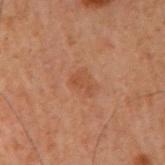workup: no biopsy performed (imaged during a skin exam) | illumination: cross-polarized illumination | anatomic site: the right upper arm | acquisition: total-body-photography crop, ~15 mm field of view | lesion diameter: ~2.5 mm (longest diameter) | image-analysis metrics: a mean CIELAB color near L≈37 a*≈20 b*≈27, about 5 CIELAB-L* units darker than the surrounding skin, and a lesion-to-skin contrast of about 5 (normalized; higher = more distinct); a nevus-likeness score of about 0/100 and a lesion-detection confidence of about 100/100 | patient: male, approximately 60 years of age.